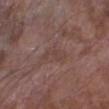The lesion was tiled from a total-body skin photograph and was not biopsied.
Approximately 3.5 mm at its widest.
From the left lower leg.
The tile uses white-light illumination.
Cropped from a total-body skin-imaging series; the visible field is about 15 mm.
A male patient aged 78–82.